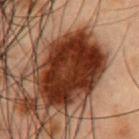Part of a total-body skin-imaging series; this lesion was reviewed on a skin check and was not flagged for biopsy.
A lesion tile, about 15 mm wide, cut from a 3D total-body photograph.
A male subject, in their mid-50s.
The lesion is on the chest.
The lesion-visualizer software estimated a footprint of about 45 mm², an outline eccentricity of about 0.75 (0 = round, 1 = elongated), and a shape-asymmetry score of about 0.15 (0 = symmetric). The software also gave a classifier nevus-likeness of about 65/100 and a detector confidence of about 100 out of 100 that the crop contains a lesion.
Captured under cross-polarized illumination.
Measured at roughly 9.5 mm in maximum diameter.